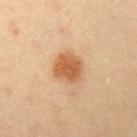Notes:
– notes: catalogued during a skin exam; not biopsied
– imaging modality: total-body-photography crop, ~15 mm field of view
– lesion diameter: about 3.5 mm
– subject: male, aged 33 to 37
– lighting: cross-polarized
– body site: the arm